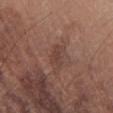No biopsy was performed on this lesion — it was imaged during a full skin examination and was not determined to be concerning.
The subject is a male aged 68 to 72.
An algorithmic analysis of the crop reported a shape eccentricity near 0.85 and a symmetry-axis asymmetry near 0.45. It also reported an automated nevus-likeness rating near 0 out of 100 and lesion-presence confidence of about 100/100.
Imaged with white-light lighting.
On the right forearm.
The lesion's longest dimension is about 2.5 mm.
Cropped from a whole-body photographic skin survey; the tile spans about 15 mm.